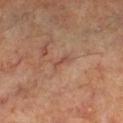The lesion was photographed on a routine skin check and not biopsied; there is no pathology result. About 2.5 mm across. The total-body-photography lesion software estimated a lesion area of about 2 mm², a shape eccentricity near 0.95, and a shape-asymmetry score of about 0.35 (0 = symmetric). And it measured border irregularity of about 4 on a 0–10 scale and a within-lesion color-variation index near 0/10. A 15 mm close-up tile from a total-body photography series done for melanoma screening. Imaged with cross-polarized lighting. A male subject roughly 60 years of age. The lesion is located on the left lower leg.